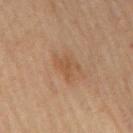<record>
<image>
  <source>total-body photography crop</source>
  <field_of_view_mm>15</field_of_view_mm>
</image>
<patient>
  <sex>male</sex>
  <age_approx>85+</age_approx>
</patient>
<lighting>cross-polarized</lighting>
<site>arm</site>
</record>